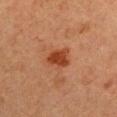The lesion is located on the right upper arm.
A 15 mm close-up extracted from a 3D total-body photography capture.
A female subject approximately 40 years of age.